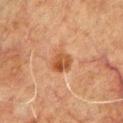This lesion was catalogued during total-body skin photography and was not selected for biopsy. Cropped from a total-body skin-imaging series; the visible field is about 15 mm. Imaged with cross-polarized lighting. A male subject, aged approximately 50. On the chest. Measured at roughly 3 mm in maximum diameter.